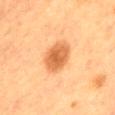Image and clinical context: About 4.5 mm across. The lesion is located on the mid back. An algorithmic analysis of the crop reported a mean CIELAB color near L≈52 a*≈23 b*≈38, a lesion–skin lightness drop of about 12, and a normalized border contrast of about 8. The analysis additionally found a border-irregularity index near 1.5/10, internal color variation of about 3 on a 0–10 scale, and peripheral color asymmetry of about 0.5. It also reported an automated nevus-likeness rating near 100 out of 100 and a detector confidence of about 100 out of 100 that the crop contains a lesion. This image is a 15 mm lesion crop taken from a total-body photograph. The subject is a female aged 53 to 57.